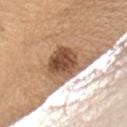biopsy status: total-body-photography surveillance lesion; no biopsy | subject: female, aged around 60 | lighting: white-light | anatomic site: the left upper arm | acquisition: total-body-photography crop, ~15 mm field of view.